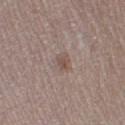{
  "biopsy_status": "not biopsied; imaged during a skin examination",
  "automated_metrics": {
    "area_mm2_approx": 4.0,
    "eccentricity": 0.7,
    "shape_asymmetry": 0.25,
    "vs_skin_darker_L": 7.0,
    "vs_skin_contrast_norm": 5.5,
    "border_irregularity_0_10": 2.5,
    "color_variation_0_10": 3.0
  },
  "lighting": "white-light",
  "patient": {
    "sex": "female",
    "age_approx": 40
  },
  "image": {
    "source": "total-body photography crop",
    "field_of_view_mm": 15
  },
  "lesion_size": {
    "long_diameter_mm_approx": 2.5
  },
  "site": "right thigh"
}